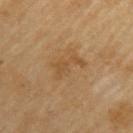Imaged during a routine full-body skin examination; the lesion was not biopsied and no histopathology is available. The total-body-photography lesion software estimated a shape eccentricity near 0.9 and a symmetry-axis asymmetry near 0.55. The software also gave about 6 CIELAB-L* units darker than the surrounding skin and a lesion-to-skin contrast of about 5.5 (normalized; higher = more distinct). And it measured a border-irregularity rating of about 7.5/10 and radial color variation of about 0.5. The analysis additionally found a classifier nevus-likeness of about 0/100 and a detector confidence of about 100 out of 100 that the crop contains a lesion. The lesion is on the arm. Cropped from a whole-body photographic skin survey; the tile spans about 15 mm. A male subject, roughly 70 years of age. The recorded lesion diameter is about 4 mm. Imaged with cross-polarized lighting.